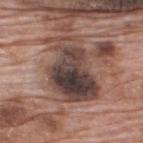This lesion was catalogued during total-body skin photography and was not selected for biopsy.
Automated tile analysis of the lesion measured a classifier nevus-likeness of about 0/100 and lesion-presence confidence of about 100/100.
Measured at roughly 7.5 mm in maximum diameter.
A 15 mm close-up tile from a total-body photography series done for melanoma screening.
A male subject about 70 years old.
Imaged with white-light lighting.
The lesion is on the upper back.